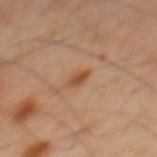  site: mid back
  lighting: cross-polarized
  lesion_size:
    long_diameter_mm_approx: 2.5
  patient:
    sex: male
    age_approx: 45
  image:
    source: total-body photography crop
    field_of_view_mm: 15
  automated_metrics:
    cielab_L: 47
    cielab_a: 20
    cielab_b: 33
    vs_skin_darker_L: 9.0
    vs_skin_contrast_norm: 7.5
    border_irregularity_0_10: 2.5
    color_variation_0_10: 1.5
    peripheral_color_asymmetry: 0.5
    nevus_likeness_0_100: 65
    lesion_detection_confidence_0_100: 100